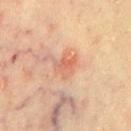Case summary:
• biopsy status · catalogued during a skin exam; not biopsied
• TBP lesion metrics · a lesion area of about 5.5 mm², a shape eccentricity near 0.55, and two-axis asymmetry of about 0.3
• location · the chest
• lighting · cross-polarized illumination
• acquisition · total-body-photography crop, ~15 mm field of view
• lesion diameter · ≈3 mm
• subject · male, aged approximately 65